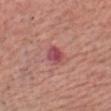A male subject in their 70s. The lesion is located on the head or neck. This is a white-light tile. Approximately 2.5 mm at its widest. Automated tile analysis of the lesion measured an average lesion color of about L≈49 a*≈32 b*≈20 (CIELAB) and a normalized border contrast of about 8.5. And it measured a border-irregularity index near 2/10, a within-lesion color-variation index near 4.5/10, and peripheral color asymmetry of about 1.5. And it measured lesion-presence confidence of about 100/100. A lesion tile, about 15 mm wide, cut from a 3D total-body photograph.